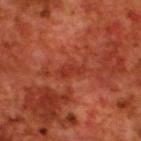Q: Was this lesion biopsied?
A: catalogued during a skin exam; not biopsied
Q: Illumination type?
A: cross-polarized illumination
Q: How large is the lesion?
A: about 3 mm
Q: Where on the body is the lesion?
A: the upper back
Q: What are the patient's age and sex?
A: male, aged 68–72
Q: What kind of image is this?
A: total-body-photography crop, ~15 mm field of view